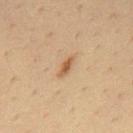Clinical impression: The lesion was tiled from a total-body skin photograph and was not biopsied. Clinical summary: Measured at roughly 3 mm in maximum diameter. A lesion tile, about 15 mm wide, cut from a 3D total-body photograph. This is a cross-polarized tile. The lesion is on the mid back. The subject is a male about 35 years old. An algorithmic analysis of the crop reported a footprint of about 2.5 mm², an outline eccentricity of about 0.9 (0 = round, 1 = elongated), and a symmetry-axis asymmetry near 0.25. The analysis additionally found a mean CIELAB color near L≈52 a*≈18 b*≈34 and about 11 CIELAB-L* units darker than the surrounding skin. The software also gave a classifier nevus-likeness of about 70/100.